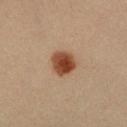Imaged during a routine full-body skin examination; the lesion was not biopsied and no histopathology is available. On the right forearm. The total-body-photography lesion software estimated a lesion area of about 7.5 mm² and a shape eccentricity near 0.4. It also reported border irregularity of about 1.5 on a 0–10 scale, a color-variation rating of about 4.5/10, and radial color variation of about 1.5. It also reported an automated nevus-likeness rating near 100 out of 100 and lesion-presence confidence of about 100/100. Captured under cross-polarized illumination. A 15 mm close-up tile from a total-body photography series done for melanoma screening. The subject is a male aged approximately 35.